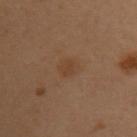<tbp_lesion>
<biopsy_status>not biopsied; imaged during a skin examination</biopsy_status>
<automated_metrics>
  <border_irregularity_0_10>2.0</border_irregularity_0_10>
  <peripheral_color_asymmetry>0.5</peripheral_color_asymmetry>
  <nevus_likeness_0_100>10</nevus_likeness_0_100>
  <lesion_detection_confidence_0_100>100</lesion_detection_confidence_0_100>
</automated_metrics>
<image>
  <source>total-body photography crop</source>
  <field_of_view_mm>15</field_of_view_mm>
</image>
<patient>
  <sex>female</sex>
  <age_approx>50</age_approx>
</patient>
<site>chest</site>
<lesion_size>
  <long_diameter_mm_approx>2.5</long_diameter_mm_approx>
</lesion_size>
<lighting>cross-polarized</lighting>
</tbp_lesion>